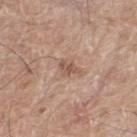notes: catalogued during a skin exam; not biopsied
patient: male, approximately 70 years of age
image source: 15 mm crop, total-body photography
automated lesion analysis: a footprint of about 3.5 mm², a shape eccentricity near 0.8, and a shape-asymmetry score of about 0.35 (0 = symmetric); a lesion color around L≈54 a*≈19 b*≈28 in CIELAB and a lesion–skin lightness drop of about 9; a border-irregularity rating of about 3.5/10 and a within-lesion color-variation index near 1.5/10; lesion-presence confidence of about 100/100
lighting: white-light illumination
location: the right thigh
diameter: ~3 mm (longest diameter)